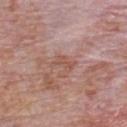Assessment:
Recorded during total-body skin imaging; not selected for excision or biopsy.
Background:
A male subject roughly 80 years of age. Captured under white-light illumination. From the chest. The total-body-photography lesion software estimated an area of roughly 4 mm² and two-axis asymmetry of about 0.5. It also reported an average lesion color of about L≈53 a*≈22 b*≈26 (CIELAB), about 7 CIELAB-L* units darker than the surrounding skin, and a lesion-to-skin contrast of about 5.5 (normalized; higher = more distinct). And it measured border irregularity of about 6 on a 0–10 scale, internal color variation of about 1 on a 0–10 scale, and a peripheral color-asymmetry measure near 0.5. A 15 mm close-up extracted from a 3D total-body photography capture.